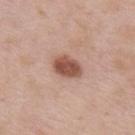This lesion was catalogued during total-body skin photography and was not selected for biopsy. From the chest. A 15 mm close-up tile from a total-body photography series done for melanoma screening. The lesion's longest dimension is about 3.5 mm. This is a white-light tile. The subject is a male about 55 years old.